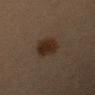Context:
Cropped from a total-body skin-imaging series; the visible field is about 15 mm. The patient is a female roughly 40 years of age. Measured at roughly 4.5 mm in maximum diameter. Automated image analysis of the tile measured an automated nevus-likeness rating near 100 out of 100. The lesion is located on the left upper arm.